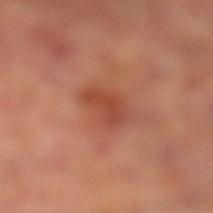The lesion was photographed on a routine skin check and not biopsied; there is no pathology result.
Imaged with cross-polarized lighting.
A lesion tile, about 15 mm wide, cut from a 3D total-body photograph.
Longest diameter approximately 4 mm.
From the left lower leg.
The subject is a male aged around 65.